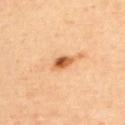follow-up: catalogued during a skin exam; not biopsied | site: the upper back | patient: male, aged approximately 40 | acquisition: total-body-photography crop, ~15 mm field of view | tile lighting: cross-polarized.